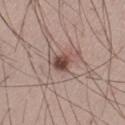<case>
  <biopsy_status>not biopsied; imaged during a skin examination</biopsy_status>
  <lesion_size>
    <long_diameter_mm_approx>2.5</long_diameter_mm_approx>
  </lesion_size>
  <patient>
    <sex>male</sex>
    <age_approx>30</age_approx>
  </patient>
  <site>left thigh</site>
  <image>
    <source>total-body photography crop</source>
    <field_of_view_mm>15</field_of_view_mm>
  </image>
</case>